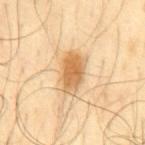workup: total-body-photography surveillance lesion; no biopsy
patient: male, in their mid-60s
lesion diameter: about 4.5 mm
anatomic site: the mid back
illumination: cross-polarized
TBP lesion metrics: a lesion area of about 11 mm² and an eccentricity of roughly 0.8; a mean CIELAB color near L≈63 a*≈18 b*≈40, a lesion–skin lightness drop of about 13, and a lesion-to-skin contrast of about 8.5 (normalized; higher = more distinct); a border-irregularity rating of about 2/10, a color-variation rating of about 4/10, and radial color variation of about 1.5; a classifier nevus-likeness of about 95/100 and a detector confidence of about 100 out of 100 that the crop contains a lesion
image: ~15 mm tile from a whole-body skin photo